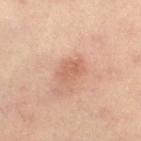| key | value |
|---|---|
| image | ~15 mm crop, total-body skin-cancer survey |
| patient | male, in their mid-60s |
| TBP lesion metrics | an outline eccentricity of about 0.75 (0 = round, 1 = elongated) and a shape-asymmetry score of about 0.25 (0 = symmetric); a lesion color around L≈58 a*≈22 b*≈30 in CIELAB and roughly 8 lightness units darker than nearby skin; a border-irregularity index near 3/10, a within-lesion color-variation index near 2.5/10, and radial color variation of about 1; a lesion-detection confidence of about 100/100 |
| site | the right thigh |
| lighting | cross-polarized illumination |